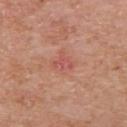A 15 mm close-up extracted from a 3D total-body photography capture. On the upper back. The total-body-photography lesion software estimated an outline eccentricity of about 0.75 (0 = round, 1 = elongated) and a shape-asymmetry score of about 0.6 (0 = symmetric). It also reported a lesion color around L≈54 a*≈30 b*≈28 in CIELAB, a lesion–skin lightness drop of about 7, and a lesion-to-skin contrast of about 5 (normalized; higher = more distinct). The software also gave a border-irregularity index near 6/10, a color-variation rating of about 1.5/10, and radial color variation of about 0.5. And it measured an automated nevus-likeness rating near 0 out of 100. The subject is a male roughly 75 years of age. Approximately 2.5 mm at its widest.